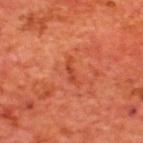Impression: The lesion was photographed on a routine skin check and not biopsied; there is no pathology result. Image and clinical context: A male patient, aged around 65. A close-up tile cropped from a whole-body skin photograph, about 15 mm across. The lesion-visualizer software estimated roughly 7 lightness units darker than nearby skin and a lesion-to-skin contrast of about 5.5 (normalized; higher = more distinct). The lesion is on the upper back. Imaged with cross-polarized lighting. Approximately 3 mm at its widest.